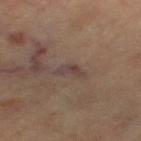{
  "biopsy_status": "not biopsied; imaged during a skin examination",
  "lighting": "cross-polarized",
  "site": "left thigh",
  "patient": {
    "age_approx": 60
  },
  "automated_metrics": {
    "area_mm2_approx": 3.5,
    "shape_asymmetry": 0.5,
    "cielab_L": 40,
    "cielab_a": 15,
    "cielab_b": 18,
    "vs_skin_darker_L": 7.0,
    "vs_skin_contrast_norm": 7.5,
    "border_irregularity_0_10": 5.0,
    "color_variation_0_10": 0.0,
    "nevus_likeness_0_100": 0,
    "lesion_detection_confidence_0_100": 55
  },
  "image": {
    "source": "total-body photography crop",
    "field_of_view_mm": 15
  }
}